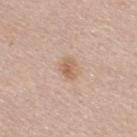No biopsy was performed on this lesion — it was imaged during a full skin examination and was not determined to be concerning. Automated tile analysis of the lesion measured border irregularity of about 2 on a 0–10 scale, internal color variation of about 3 on a 0–10 scale, and peripheral color asymmetry of about 1. Located on the upper back. The lesion's longest dimension is about 2.5 mm. A roughly 15 mm field-of-view crop from a total-body skin photograph. A male subject, aged approximately 30. The tile uses white-light illumination.